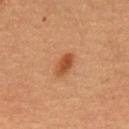| key | value |
|---|---|
| notes | no biopsy performed (imaged during a skin exam) |
| subject | male, in their 50s |
| image source | 15 mm crop, total-body photography |
| illumination | cross-polarized |
| site | the abdomen |
| lesion diameter | ~3 mm (longest diameter) |